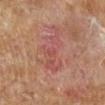The lesion was tiled from a total-body skin photograph and was not biopsied.
A 15 mm close-up tile from a total-body photography series done for melanoma screening.
On the leg.
The patient is a male aged 58 to 62.